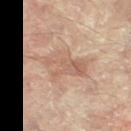Assessment: Part of a total-body skin-imaging series; this lesion was reviewed on a skin check and was not flagged for biopsy. Acquisition and patient details: Measured at roughly 6 mm in maximum diameter. Located on the left leg. A female subject about 80 years old. Captured under cross-polarized illumination. A 15 mm close-up extracted from a 3D total-body photography capture.